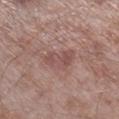Notes:
– biopsy status · catalogued during a skin exam; not biopsied
– patient · male, aged 53–57
– acquisition · total-body-photography crop, ~15 mm field of view
– site · the leg
– automated metrics · a mean CIELAB color near L≈50 a*≈21 b*≈21, about 8 CIELAB-L* units darker than the surrounding skin, and a normalized border contrast of about 6; a nevus-likeness score of about 0/100 and a lesion-detection confidence of about 100/100